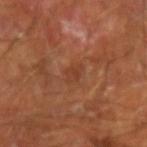workup=no biopsy performed (imaged during a skin exam); lighting=cross-polarized illumination; subject=male, about 65 years old; image source=total-body-photography crop, ~15 mm field of view; location=the left forearm; diameter=≈2.5 mm.